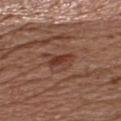Part of a total-body skin-imaging series; this lesion was reviewed on a skin check and was not flagged for biopsy.
From the chest.
The recorded lesion diameter is about 3 mm.
Captured under white-light illumination.
The patient is a male aged around 65.
A 15 mm close-up extracted from a 3D total-body photography capture.